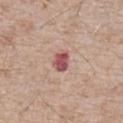  biopsy_status: not biopsied; imaged during a skin examination
  image:
    source: total-body photography crop
    field_of_view_mm: 15
  automated_metrics:
    cielab_L: 53
    cielab_a: 28
    cielab_b: 22
    vs_skin_darker_L: 14.0
    vs_skin_contrast_norm: 10.0
    border_irregularity_0_10: 2.0
    color_variation_0_10: 3.5
    peripheral_color_asymmetry: 1.0
    nevus_likeness_0_100: 0
    lesion_detection_confidence_0_100: 100
  lesion_size:
    long_diameter_mm_approx: 2.5
  patient:
    sex: male
    age_approx: 65
  site: chest
  lighting: white-light